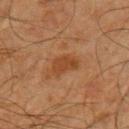Assessment:
Captured during whole-body skin photography for melanoma surveillance; the lesion was not biopsied.
Clinical summary:
A male subject in their mid- to late 60s. The lesion is located on the arm. The recorded lesion diameter is about 3.5 mm. A 15 mm close-up extracted from a 3D total-body photography capture. Automated image analysis of the tile measured an area of roughly 6.5 mm² and two-axis asymmetry of about 0.3. The software also gave a border-irregularity rating of about 3/10, a color-variation rating of about 2/10, and a peripheral color-asymmetry measure near 0.5.